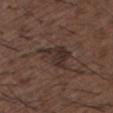workup: no biopsy performed (imaged during a skin exam) | TBP lesion metrics: an eccentricity of roughly 0.85 and a shape-asymmetry score of about 0.5 (0 = symmetric); an automated nevus-likeness rating near 15 out of 100 and a detector confidence of about 80 out of 100 that the crop contains a lesion | image source: ~15 mm tile from a whole-body skin photo | tile lighting: white-light | lesion diameter: ≈5.5 mm | subject: male, roughly 50 years of age | body site: the upper back.